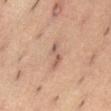This lesion was catalogued during total-body skin photography and was not selected for biopsy.
A close-up tile cropped from a whole-body skin photograph, about 15 mm across.
On the front of the torso.
About 3.5 mm across.
A male patient, aged 53–57.
Captured under cross-polarized illumination.